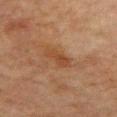<tbp_lesion>
  <lighting>cross-polarized</lighting>
  <lesion_size>
    <long_diameter_mm_approx>3.0</long_diameter_mm_approx>
  </lesion_size>
  <image>
    <source>total-body photography crop</source>
    <field_of_view_mm>15</field_of_view_mm>
  </image>
  <site>abdomen</site>
  <patient>
    <sex>male</sex>
    <age_approx>80</age_approx>
  </patient>
  <automated_metrics>
    <area_mm2_approx>5.0</area_mm2_approx>
    <eccentricity>0.8</eccentricity>
    <shape_asymmetry>0.25</shape_asymmetry>
    <cielab_L>35</cielab_L>
    <cielab_a>18</cielab_a>
    <cielab_b>29</cielab_b>
    <vs_skin_darker_L>6.0</vs_skin_darker_L>
    <vs_skin_contrast_norm>6.0</vs_skin_contrast_norm>
    <nevus_likeness_0_100>0</nevus_likeness_0_100>
    <lesion_detection_confidence_0_100>100</lesion_detection_confidence_0_100>
  </automated_metrics>
</tbp_lesion>